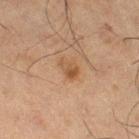Captured during whole-body skin photography for melanoma surveillance; the lesion was not biopsied. On the right thigh. Cropped from a whole-body photographic skin survey; the tile spans about 15 mm. A male subject, in their mid- to late 60s.